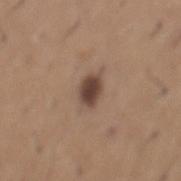Clinical impression:
Imaged during a routine full-body skin examination; the lesion was not biopsied and no histopathology is available.
Clinical summary:
The lesion is located on the mid back. The patient is a male approximately 65 years of age. A 15 mm close-up extracted from a 3D total-body photography capture.